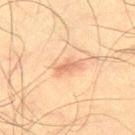notes: catalogued during a skin exam; not biopsied
patient: male, aged around 50
lighting: cross-polarized
site: the right thigh
image: 15 mm crop, total-body photography